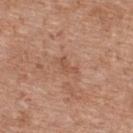{"biopsy_status": "not biopsied; imaged during a skin examination", "automated_metrics": {"area_mm2_approx": 3.0, "eccentricity": 0.85, "shape_asymmetry": 0.45, "nevus_likeness_0_100": 0, "lesion_detection_confidence_0_100": 100}, "image": {"source": "total-body photography crop", "field_of_view_mm": 15}, "lighting": "white-light", "patient": {"sex": "female", "age_approx": 40}, "lesion_size": {"long_diameter_mm_approx": 3.0}, "site": "upper back"}